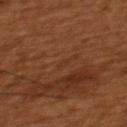Imaged during a routine full-body skin examination; the lesion was not biopsied and no histopathology is available. Located on the upper back. The subject is a male aged around 45. A 15 mm close-up extracted from a 3D total-body photography capture.